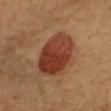workup: no biopsy performed (imaged during a skin exam)
diameter: ≈6.5 mm
anatomic site: the right upper arm
image source: total-body-photography crop, ~15 mm field of view
image-analysis metrics: a footprint of about 23 mm², an outline eccentricity of about 0.7 (0 = round, 1 = elongated), and a shape-asymmetry score of about 0.15 (0 = symmetric); a border-irregularity index near 2/10, a within-lesion color-variation index near 5.5/10, and a peripheral color-asymmetry measure near 2; an automated nevus-likeness rating near 100 out of 100 and a lesion-detection confidence of about 100/100
tile lighting: cross-polarized illumination
patient: female, about 40 years old